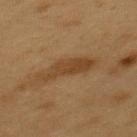Acquisition and patient details: On the mid back. Captured under cross-polarized illumination. The subject is a male aged 53–57. The total-body-photography lesion software estimated an average lesion color of about L≈37 a*≈17 b*≈31 (CIELAB), a lesion–skin lightness drop of about 7, and a lesion-to-skin contrast of about 7 (normalized; higher = more distinct). And it measured a nevus-likeness score of about 25/100. The lesion's longest dimension is about 6 mm. A region of skin cropped from a whole-body photographic capture, roughly 15 mm wide.